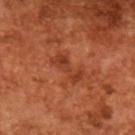Captured during whole-body skin photography for melanoma surveillance; the lesion was not biopsied. Approximately 4 mm at its widest. An algorithmic analysis of the crop reported an average lesion color of about L≈39 a*≈30 b*≈35 (CIELAB), a lesion–skin lightness drop of about 8, and a lesion-to-skin contrast of about 6 (normalized; higher = more distinct). It also reported a border-irregularity rating of about 6.5/10, internal color variation of about 2 on a 0–10 scale, and radial color variation of about 0.5. This is a cross-polarized tile. A male patient aged approximately 65. A 15 mm crop from a total-body photograph taken for skin-cancer surveillance.